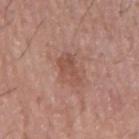Q: Patient demographics?
A: male, aged 68 to 72
Q: What is the imaging modality?
A: ~15 mm tile from a whole-body skin photo
Q: What is the lesion's diameter?
A: about 3.5 mm
Q: Illumination type?
A: white-light illumination
Q: Lesion location?
A: the right upper arm